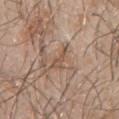<record>
  <site>chest</site>
  <image>
    <source>total-body photography crop</source>
    <field_of_view_mm>15</field_of_view_mm>
  </image>
  <patient>
    <sex>male</sex>
    <age_approx>30</age_approx>
  </patient>
  <lesion_size>
    <long_diameter_mm_approx>3.0</long_diameter_mm_approx>
  </lesion_size>
</record>